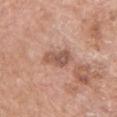– biopsy status — total-body-photography surveillance lesion; no biopsy
– imaging modality — ~15 mm tile from a whole-body skin photo
– site — the front of the torso
– patient — female, aged 58–62